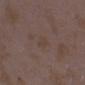  biopsy_status: not biopsied; imaged during a skin examination
  patient:
    sex: female
    age_approx: 35
  site: right forearm
  image:
    source: total-body photography crop
    field_of_view_mm: 15
  automated_metrics:
    area_mm2_approx: 2.5
    eccentricity: 0.9
    shape_asymmetry: 0.3
    color_variation_0_10: 0.0
    nevus_likeness_0_100: 0
    lesion_detection_confidence_0_100: 100